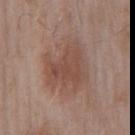<lesion>
<biopsy_status>not biopsied; imaged during a skin examination</biopsy_status>
<image>
  <source>total-body photography crop</source>
  <field_of_view_mm>15</field_of_view_mm>
</image>
<lighting>white-light</lighting>
<patient>
  <sex>male</sex>
  <age_approx>55</age_approx>
</patient>
<site>right upper arm</site>
<lesion_size>
  <long_diameter_mm_approx>6.0</long_diameter_mm_approx>
</lesion_size>
</lesion>